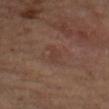Clinical summary: A female subject, in their 70s. Cropped from a total-body skin-imaging series; the visible field is about 15 mm. The lesion is on the left forearm.